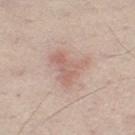Part of a total-body skin-imaging series; this lesion was reviewed on a skin check and was not flagged for biopsy. This is a white-light tile. A male subject approximately 65 years of age. Cropped from a whole-body photographic skin survey; the tile spans about 15 mm. On the left thigh. Longest diameter approximately 4.5 mm.